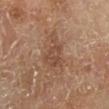This lesion was catalogued during total-body skin photography and was not selected for biopsy. A 15 mm close-up tile from a total-body photography series done for melanoma screening. Imaged with cross-polarized lighting. A male patient, aged approximately 65. From the right lower leg.